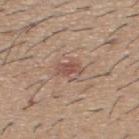Imaged during a routine full-body skin examination; the lesion was not biopsied and no histopathology is available. A male subject, approximately 60 years of age. From the upper back. The recorded lesion diameter is about 3 mm. Imaged with white-light lighting. A 15 mm close-up tile from a total-body photography series done for melanoma screening.